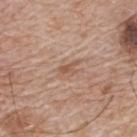Clinical impression:
Imaged during a routine full-body skin examination; the lesion was not biopsied and no histopathology is available.
Image and clinical context:
The patient is a male aged 63 to 67. On the back. Imaged with white-light lighting. A 15 mm close-up extracted from a 3D total-body photography capture.